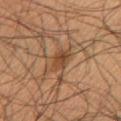Assessment: Imaged during a routine full-body skin examination; the lesion was not biopsied and no histopathology is available. Clinical summary: A male subject, approximately 35 years of age. This image is a 15 mm lesion crop taken from a total-body photograph. The tile uses cross-polarized illumination. From the right forearm. Longest diameter approximately 3 mm.